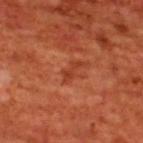follow-up: imaged on a skin check; not biopsied
TBP lesion metrics: a footprint of about 2.5 mm², an eccentricity of roughly 0.9, and two-axis asymmetry of about 0.5; a lesion color around L≈40 a*≈32 b*≈36 in CIELAB and a normalized lesion–skin contrast near 6
patient: male, aged 68 to 72
site: the upper back
lighting: cross-polarized
image: ~15 mm crop, total-body skin-cancer survey
lesion size: ~3 mm (longest diameter)